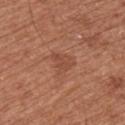<record>
<biopsy_status>not biopsied; imaged during a skin examination</biopsy_status>
<lighting>white-light</lighting>
<image>
  <source>total-body photography crop</source>
  <field_of_view_mm>15</field_of_view_mm>
</image>
<patient>
  <sex>male</sex>
  <age_approx>65</age_approx>
</patient>
<site>arm</site>
<lesion_size>
  <long_diameter_mm_approx>3.0</long_diameter_mm_approx>
</lesion_size>
</record>